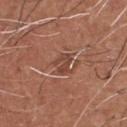Part of a total-body skin-imaging series; this lesion was reviewed on a skin check and was not flagged for biopsy.
A 15 mm close-up tile from a total-body photography series done for melanoma screening.
The recorded lesion diameter is about 2.5 mm.
The patient is a male aged 63–67.
The lesion is on the chest.
The total-body-photography lesion software estimated a footprint of about 4 mm² and an eccentricity of roughly 0.7. It also reported a lesion color around L≈44 a*≈23 b*≈28 in CIELAB and about 8 CIELAB-L* units darker than the surrounding skin. The analysis additionally found a within-lesion color-variation index near 3.5/10 and radial color variation of about 1.5.